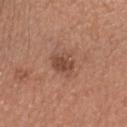Recorded during total-body skin imaging; not selected for excision or biopsy.
Automated tile analysis of the lesion measured a mean CIELAB color near L≈47 a*≈22 b*≈28, a lesion–skin lightness drop of about 10, and a normalized lesion–skin contrast near 7.5.
Captured under white-light illumination.
Located on the head or neck.
A lesion tile, about 15 mm wide, cut from a 3D total-body photograph.
The subject is a female about 30 years old.
Measured at roughly 3 mm in maximum diameter.